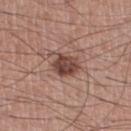Impression: This lesion was catalogued during total-body skin photography and was not selected for biopsy. Context: Automated image analysis of the tile measured a lesion area of about 7 mm², an outline eccentricity of about 0.65 (0 = round, 1 = elongated), and a symmetry-axis asymmetry near 0.25. The analysis additionally found a lesion color around L≈44 a*≈20 b*≈23 in CIELAB, about 14 CIELAB-L* units darker than the surrounding skin, and a normalized border contrast of about 10.5. The software also gave a classifier nevus-likeness of about 85/100 and lesion-presence confidence of about 100/100. The lesion is on the right lower leg. This is a white-light tile. A male subject, aged 58 to 62. Cropped from a total-body skin-imaging series; the visible field is about 15 mm. About 3.5 mm across.